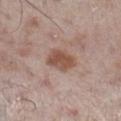Q: Was a biopsy performed?
A: imaged on a skin check; not biopsied
Q: What are the patient's age and sex?
A: male, aged around 60
Q: Automated lesion metrics?
A: a footprint of about 7.5 mm², an outline eccentricity of about 0.75 (0 = round, 1 = elongated), and a symmetry-axis asymmetry near 0.2; a border-irregularity rating of about 2/10 and radial color variation of about 1
Q: How large is the lesion?
A: ≈3.5 mm
Q: What is the anatomic site?
A: the right lower leg
Q: How was the tile lit?
A: white-light
Q: What is the imaging modality?
A: ~15 mm crop, total-body skin-cancer survey